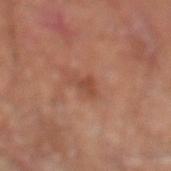biopsy status — imaged on a skin check; not biopsied
patient — male, aged 68 to 72
lesion diameter — about 2.5 mm
illumination — cross-polarized illumination
image source — ~15 mm crop, total-body skin-cancer survey
anatomic site — the left lower leg